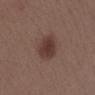follow-up=imaged on a skin check; not biopsied
body site=the right lower leg
acquisition=total-body-photography crop, ~15 mm field of view
lighting=white-light illumination
lesion size=about 4 mm
subject=female, about 40 years old